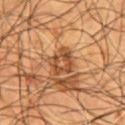workup: catalogued during a skin exam; not biopsied | patient: male, about 55 years old | size: about 3.5 mm | imaging modality: 15 mm crop, total-body photography | body site: the chest.